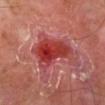| field | value |
|---|---|
| notes | no biopsy performed (imaged during a skin exam) |
| automated lesion analysis | a lesion area of about 17 mm² and an eccentricity of roughly 0.8; a mean CIELAB color near L≈41 a*≈37 b*≈28, roughly 11 lightness units darker than nearby skin, and a lesion-to-skin contrast of about 9.5 (normalized; higher = more distinct); a border-irregularity index near 4.5/10, a color-variation rating of about 8/10, and peripheral color asymmetry of about 2.5; a classifier nevus-likeness of about 35/100 |
| subject | male, roughly 65 years of age |
| diameter | ≈6 mm |
| acquisition | ~15 mm crop, total-body skin-cancer survey |